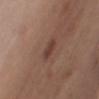workup — total-body-photography surveillance lesion; no biopsy
subject — female, aged around 40
body site — the front of the torso
image source — ~15 mm crop, total-body skin-cancer survey
automated metrics — a footprint of about 4.5 mm², a shape eccentricity near 0.9, and two-axis asymmetry of about 0.25; border irregularity of about 3 on a 0–10 scale, a color-variation rating of about 1.5/10, and radial color variation of about 0.5
lesion size — about 3.5 mm
tile lighting — white-light